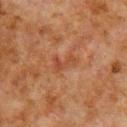Q: Lesion size?
A: ≈2.5 mm
Q: Lesion location?
A: the arm
Q: What did automated image analysis measure?
A: a mean CIELAB color near L≈36 a*≈22 b*≈28, a lesion–skin lightness drop of about 6, and a lesion-to-skin contrast of about 5.5 (normalized; higher = more distinct); a border-irregularity rating of about 7.5/10, a color-variation rating of about 0/10, and a peripheral color-asymmetry measure near 0
Q: What is the imaging modality?
A: 15 mm crop, total-body photography
Q: What are the patient's age and sex?
A: male, about 80 years old